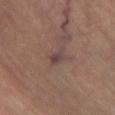Clinical summary:
The lesion is on the left lower leg. A close-up tile cropped from a whole-body skin photograph, about 15 mm across. A female subject, roughly 65 years of age.